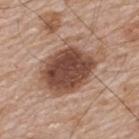Impression:
The lesion was tiled from a total-body skin photograph and was not biopsied.
Context:
Located on the upper back. Cropped from a whole-body photographic skin survey; the tile spans about 15 mm. A male patient, aged 63 to 67. About 7.5 mm across. Captured under white-light illumination.